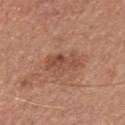* notes: imaged on a skin check; not biopsied
* acquisition: 15 mm crop, total-body photography
* automated lesion analysis: a lesion area of about 7.5 mm² and a symmetry-axis asymmetry near 0.3; an automated nevus-likeness rating near 0 out of 100 and a lesion-detection confidence of about 100/100
* tile lighting: white-light illumination
* location: the mid back
* patient: male, aged 63–67